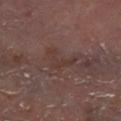workup: no biopsy performed (imaged during a skin exam) | automated lesion analysis: an average lesion color of about L≈34 a*≈17 b*≈20 (CIELAB), about 5 CIELAB-L* units darker than the surrounding skin, and a normalized border contrast of about 5.5; a classifier nevus-likeness of about 0/100 and a detector confidence of about 75 out of 100 that the crop contains a lesion | subject: male, in their mid-60s | tile lighting: cross-polarized | diameter: ≈4 mm | image source: total-body-photography crop, ~15 mm field of view | location: the left lower leg.